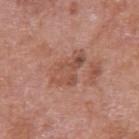Q: Automated lesion metrics?
A: a lesion area of about 9.5 mm², an outline eccentricity of about 0.85 (0 = round, 1 = elongated), and two-axis asymmetry of about 0.4; a classifier nevus-likeness of about 0/100 and a lesion-detection confidence of about 100/100
Q: How was the tile lit?
A: white-light illumination
Q: How was this image acquired?
A: total-body-photography crop, ~15 mm field of view
Q: What are the patient's age and sex?
A: male, aged around 70
Q: Lesion location?
A: the right upper arm